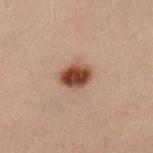Q: Is there a histopathology result?
A: no biopsy performed (imaged during a skin exam)
Q: Automated lesion metrics?
A: a footprint of about 6.5 mm², an eccentricity of roughly 0.65, and two-axis asymmetry of about 0.15; a lesion color around L≈36 a*≈19 b*≈26 in CIELAB, a lesion–skin lightness drop of about 15, and a normalized lesion–skin contrast near 13; border irregularity of about 1.5 on a 0–10 scale, internal color variation of about 4 on a 0–10 scale, and radial color variation of about 1.5; a nevus-likeness score of about 100/100
Q: How was the tile lit?
A: cross-polarized
Q: Lesion location?
A: the left lower leg
Q: How was this image acquired?
A: ~15 mm tile from a whole-body skin photo
Q: What are the patient's age and sex?
A: female, aged 38 to 42
Q: How large is the lesion?
A: about 3 mm